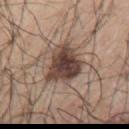Captured during whole-body skin photography for melanoma surveillance; the lesion was not biopsied. The recorded lesion diameter is about 5 mm. The patient is a male aged approximately 70. Captured under white-light illumination. A 15 mm close-up extracted from a 3D total-body photography capture. Automated tile analysis of the lesion measured a symmetry-axis asymmetry near 0.35. The software also gave a mean CIELAB color near L≈43 a*≈16 b*≈22, a lesion–skin lightness drop of about 16, and a lesion-to-skin contrast of about 12.5 (normalized; higher = more distinct). The software also gave a nevus-likeness score of about 90/100. From the arm.